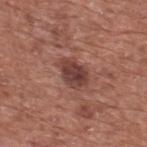follow-up: imaged on a skin check; not biopsied
lesion size: ≈4 mm
image source: ~15 mm crop, total-body skin-cancer survey
lighting: white-light illumination
subject: male, aged around 75
anatomic site: the upper back